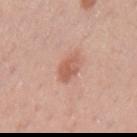Notes:
– workup · no biopsy performed (imaged during a skin exam)
– imaging modality · total-body-photography crop, ~15 mm field of view
– image-analysis metrics · a lesion area of about 5.5 mm², a shape eccentricity near 0.7, and two-axis asymmetry of about 0.25; a border-irregularity index near 2.5/10, a within-lesion color-variation index near 3.5/10, and a peripheral color-asymmetry measure near 1; a nevus-likeness score of about 75/100 and lesion-presence confidence of about 100/100
– diameter · ~3 mm (longest diameter)
– patient · male, in their mid-40s
– body site · the left upper arm